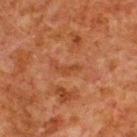Captured during whole-body skin photography for melanoma surveillance; the lesion was not biopsied. The patient is a male aged 78–82. This is a cross-polarized tile. A 15 mm close-up extracted from a 3D total-body photography capture. Located on the upper back.